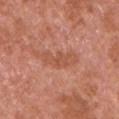Clinical summary:
Cropped from a whole-body photographic skin survey; the tile spans about 15 mm. Located on the arm. A male subject, aged 63 to 67.